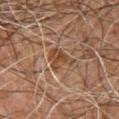workup: no biopsy performed (imaged during a skin exam)
imaging modality: total-body-photography crop, ~15 mm field of view
site: the chest
illumination: cross-polarized
automated lesion analysis: a border-irregularity index near 5/10, a color-variation rating of about 3/10, and a peripheral color-asymmetry measure near 1; a classifier nevus-likeness of about 0/100 and a lesion-detection confidence of about 60/100
patient: male, aged around 60
diameter: about 3.5 mm